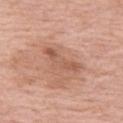Q: Is there a histopathology result?
A: catalogued during a skin exam; not biopsied
Q: What lighting was used for the tile?
A: white-light illumination
Q: What did automated image analysis measure?
A: a lesion area of about 7.5 mm² and an eccentricity of roughly 0.95
Q: What kind of image is this?
A: 15 mm crop, total-body photography
Q: What is the lesion's diameter?
A: ~5 mm (longest diameter)
Q: Patient demographics?
A: female, in their 70s
Q: What is the anatomic site?
A: the left thigh